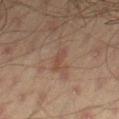<lesion>
<lighting>cross-polarized</lighting>
<automated_metrics>
  <cielab_L>37</cielab_L>
  <cielab_a>16</cielab_a>
  <cielab_b>23</cielab_b>
</automated_metrics>
<lesion_size>
  <long_diameter_mm_approx>3.0</long_diameter_mm_approx>
</lesion_size>
<site>right thigh</site>
<image>
  <source>total-body photography crop</source>
  <field_of_view_mm>15</field_of_view_mm>
</image>
<patient>
  <sex>male</sex>
  <age_approx>45</age_approx>
</patient>
</lesion>